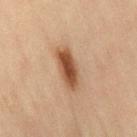workup: catalogued during a skin exam; not biopsied
location: the leg
patient: female, aged around 45
lighting: cross-polarized illumination
image source: 15 mm crop, total-body photography
lesion size: about 5 mm